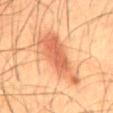Background:
Measured at roughly 8 mm in maximum diameter. The subject is a male aged 58 to 62. On the abdomen. A 15 mm close-up tile from a total-body photography series done for melanoma screening.